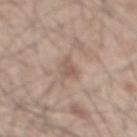follow-up: imaged on a skin check; not biopsied | patient: male, in their mid-40s | location: the mid back | automated metrics: a lesion color around L≈56 a*≈16 b*≈25 in CIELAB and a lesion–skin lightness drop of about 9; border irregularity of about 5 on a 0–10 scale and radial color variation of about 0 | lesion size: ≈3 mm | lighting: white-light | imaging modality: total-body-photography crop, ~15 mm field of view.